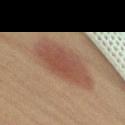Imaged with cross-polarized lighting. A female subject, in their 70s. Automated tile analysis of the lesion measured an area of roughly 21 mm² and a shape eccentricity near 0.9. The software also gave a border-irregularity rating of about 2.5/10, a within-lesion color-variation index near 3/10, and peripheral color asymmetry of about 1. And it measured an automated nevus-likeness rating near 100 out of 100 and a lesion-detection confidence of about 100/100. A 15 mm crop from a total-body photograph taken for skin-cancer surveillance. On the right thigh. The lesion's longest dimension is about 8 mm.